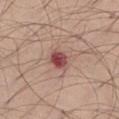Clinical summary:
Longest diameter approximately 2.5 mm. A male subject, aged approximately 25. Cropped from a total-body skin-imaging series; the visible field is about 15 mm. Captured under white-light illumination. From the leg. Automated image analysis of the tile measured a mean CIELAB color near L≈47 a*≈28 b*≈22 and a lesion-to-skin contrast of about 11 (normalized; higher = more distinct). The analysis additionally found border irregularity of about 1.5 on a 0–10 scale, a color-variation rating of about 5.5/10, and peripheral color asymmetry of about 2. It also reported a lesion-detection confidence of about 100/100.